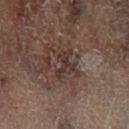This lesion was catalogued during total-body skin photography and was not selected for biopsy.
Located on the left lower leg.
The patient is a male roughly 65 years of age.
The tile uses cross-polarized illumination.
The lesion-visualizer software estimated a mean CIELAB color near L≈31 a*≈15 b*≈18, a lesion–skin lightness drop of about 7, and a normalized lesion–skin contrast near 7.5. The analysis additionally found a nevus-likeness score of about 0/100.
Approximately 3.5 mm at its widest.
Cropped from a total-body skin-imaging series; the visible field is about 15 mm.